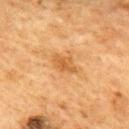Findings:
- follow-up · imaged on a skin check; not biopsied
- automated lesion analysis · a lesion area of about 4.5 mm² and a shape eccentricity near 0.8; a mean CIELAB color near L≈48 a*≈21 b*≈39, a lesion–skin lightness drop of about 8, and a normalized border contrast of about 6.5; border irregularity of about 2.5 on a 0–10 scale and radial color variation of about 1; a classifier nevus-likeness of about 10/100 and a lesion-detection confidence of about 100/100
- lesion size · about 3 mm
- image · 15 mm crop, total-body photography
- site · the upper back
- lighting · cross-polarized
- subject · female, aged 58 to 62